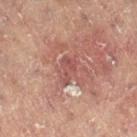Impression: The lesion was tiled from a total-body skin photograph and was not biopsied. Clinical summary: The tile uses cross-polarized illumination. A female subject, aged around 80. Cropped from a whole-body photographic skin survey; the tile spans about 15 mm. The total-body-photography lesion software estimated a footprint of about 3.5 mm², an outline eccentricity of about 0.85 (0 = round, 1 = elongated), and a shape-asymmetry score of about 0.6 (0 = symmetric). The software also gave a border-irregularity rating of about 7/10 and peripheral color asymmetry of about 1. It also reported an automated nevus-likeness rating near 0 out of 100 and a lesion-detection confidence of about 50/100. Located on the left leg.